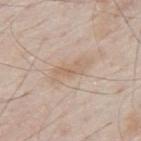biopsy status: catalogued during a skin exam; not biopsied | body site: the mid back | subject: male, aged around 80 | imaging modality: ~15 mm tile from a whole-body skin photo | tile lighting: white-light illumination | automated metrics: a footprint of about 3.5 mm² and an eccentricity of roughly 0.95; a mean CIELAB color near L≈62 a*≈16 b*≈29, a lesion–skin lightness drop of about 7, and a normalized lesion–skin contrast near 5.5; an automated nevus-likeness rating near 0 out of 100.